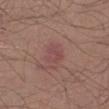biopsy status — total-body-photography surveillance lesion; no biopsy | tile lighting — white-light | subject — male, aged 23–27 | acquisition — ~15 mm tile from a whole-body skin photo | site — the left lower leg | size — about 2.5 mm.